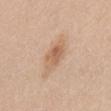Recorded during total-body skin imaging; not selected for excision or biopsy. The patient is a male aged approximately 30. This is a white-light tile. The lesion is located on the abdomen. A region of skin cropped from a whole-body photographic capture, roughly 15 mm wide.